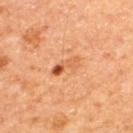The lesion was photographed on a routine skin check and not biopsied; there is no pathology result. The lesion is located on the upper back. A lesion tile, about 15 mm wide, cut from a 3D total-body photograph. A male patient, in their mid-60s. Imaged with cross-polarized lighting. Longest diameter approximately 4 mm.